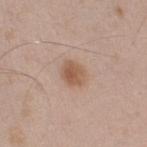Imaged during a routine full-body skin examination; the lesion was not biopsied and no histopathology is available.
The patient is a male aged around 50.
Captured under white-light illumination.
The lesion is located on the right upper arm.
This image is a 15 mm lesion crop taken from a total-body photograph.
The recorded lesion diameter is about 3 mm.